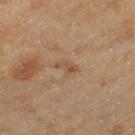workup: imaged on a skin check; not biopsied
body site: the left thigh
lesion size: ~2.5 mm (longest diameter)
subject: female, aged approximately 60
tile lighting: cross-polarized
imaging modality: ~15 mm tile from a whole-body skin photo
automated lesion analysis: a footprint of about 2.5 mm², an eccentricity of roughly 0.9, and two-axis asymmetry of about 0.4; an average lesion color of about L≈45 a*≈16 b*≈31 (CIELAB), roughly 6 lightness units darker than nearby skin, and a normalized border contrast of about 6; a classifier nevus-likeness of about 0/100 and lesion-presence confidence of about 100/100